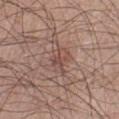This lesion was catalogued during total-body skin photography and was not selected for biopsy. On the left lower leg. The subject is a male aged 48–52. A lesion tile, about 15 mm wide, cut from a 3D total-body photograph.